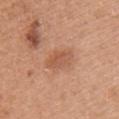Q: Was a biopsy performed?
A: no biopsy performed (imaged during a skin exam)
Q: What kind of image is this?
A: 15 mm crop, total-body photography
Q: How was the tile lit?
A: white-light
Q: Lesion size?
A: ≈4 mm
Q: What are the patient's age and sex?
A: female, aged around 40
Q: What did automated image analysis measure?
A: a classifier nevus-likeness of about 5/100 and lesion-presence confidence of about 100/100
Q: Lesion location?
A: the left upper arm